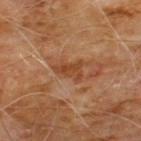The lesion was tiled from a total-body skin photograph and was not biopsied.
Longest diameter approximately 3.5 mm.
Located on the front of the torso.
A region of skin cropped from a whole-body photographic capture, roughly 15 mm wide.
A male patient, aged 58–62.